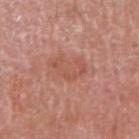The lesion was photographed on a routine skin check and not biopsied; there is no pathology result.
On the right upper arm.
Cropped from a whole-body photographic skin survey; the tile spans about 15 mm.
A male subject aged 68–72.